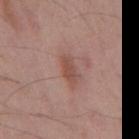notes = total-body-photography surveillance lesion; no biopsy
size = ≈3 mm
lighting = white-light illumination
acquisition = ~15 mm tile from a whole-body skin photo
patient = male, aged around 55
anatomic site = the back